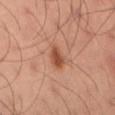Q: Is there a histopathology result?
A: catalogued during a skin exam; not biopsied
Q: What is the imaging modality?
A: 15 mm crop, total-body photography
Q: Lesion size?
A: ≈3 mm
Q: Who is the patient?
A: male, aged 48 to 52
Q: What lighting was used for the tile?
A: cross-polarized
Q: Lesion location?
A: the left thigh
Q: Automated lesion metrics?
A: about 12 CIELAB-L* units darker than the surrounding skin and a lesion-to-skin contrast of about 8.5 (normalized; higher = more distinct); a nevus-likeness score of about 95/100 and a lesion-detection confidence of about 100/100